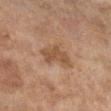{"biopsy_status": "not biopsied; imaged during a skin examination", "patient": {"sex": "female", "age_approx": 60}, "image": {"source": "total-body photography crop", "field_of_view_mm": 15}, "site": "right lower leg", "lesion_size": {"long_diameter_mm_approx": 3.5}, "lighting": "cross-polarized"}